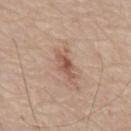site = the abdomen; image source = 15 mm crop, total-body photography; subject = male, approximately 80 years of age.